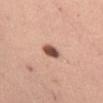Impression: Part of a total-body skin-imaging series; this lesion was reviewed on a skin check and was not flagged for biopsy. Clinical summary: The lesion is located on the left thigh. Automated tile analysis of the lesion measured a lesion area of about 4.5 mm², an outline eccentricity of about 0.55 (0 = round, 1 = elongated), and a shape-asymmetry score of about 0.2 (0 = symmetric). And it measured an average lesion color of about L≈53 a*≈22 b*≈27 (CIELAB), a lesion–skin lightness drop of about 19, and a normalized border contrast of about 12. It also reported a classifier nevus-likeness of about 100/100 and lesion-presence confidence of about 100/100. Measured at roughly 2.5 mm in maximum diameter. Cropped from a whole-body photographic skin survey; the tile spans about 15 mm. The patient is a female aged around 30. This is a white-light tile.